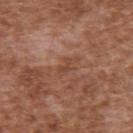Q: Was a biopsy performed?
A: total-body-photography surveillance lesion; no biopsy
Q: What are the patient's age and sex?
A: male, aged around 75
Q: How was the tile lit?
A: white-light illumination
Q: What kind of image is this?
A: total-body-photography crop, ~15 mm field of view
Q: Where on the body is the lesion?
A: the upper back